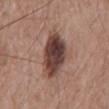| field | value |
|---|---|
| notes | catalogued during a skin exam; not biopsied |
| lesion size | about 6 mm |
| tile lighting | white-light |
| subject | male, roughly 60 years of age |
| acquisition | ~15 mm tile from a whole-body skin photo |
| site | the back |